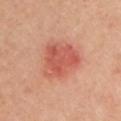notes = no biopsy performed (imaged during a skin exam); lighting = cross-polarized; site = the left upper arm; image-analysis metrics = an automated nevus-likeness rating near 5 out of 100 and a lesion-detection confidence of about 100/100; image = ~15 mm crop, total-body skin-cancer survey; subject = female, aged 58–62.